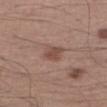Case summary:
– workup — catalogued during a skin exam; not biopsied
– patient — male, aged 53–57
– acquisition — 15 mm crop, total-body photography
– image-analysis metrics — roughly 9 lightness units darker than nearby skin and a normalized border contrast of about 7
– body site — the right upper arm
– diameter — about 2.5 mm